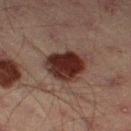This lesion was catalogued during total-body skin photography and was not selected for biopsy.
On the leg.
A male patient about 45 years old.
The tile uses cross-polarized illumination.
About 4.5 mm across.
A 15 mm close-up tile from a total-body photography series done for melanoma screening.